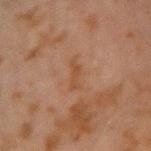Clinical impression: Recorded during total-body skin imaging; not selected for excision or biopsy. Image and clinical context: From the right upper arm. This image is a 15 mm lesion crop taken from a total-body photograph. A male subject in their mid-40s.